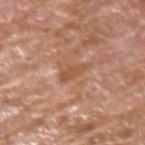Background:
Imaged with white-light lighting. The subject is a male approximately 65 years of age. A close-up tile cropped from a whole-body skin photograph, about 15 mm across. The lesion-visualizer software estimated an average lesion color of about L≈53 a*≈23 b*≈34 (CIELAB), about 8 CIELAB-L* units darker than the surrounding skin, and a lesion-to-skin contrast of about 6.5 (normalized; higher = more distinct). The lesion is located on the upper back. Longest diameter approximately 3.5 mm.